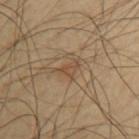The lesion was tiled from a total-body skin photograph and was not biopsied. The lesion's longest dimension is about 2.5 mm. A male subject aged 58 to 62. Automated image analysis of the tile measured a footprint of about 3 mm² and a shape-asymmetry score of about 0.5 (0 = symmetric). The lesion is located on the left upper arm. A roughly 15 mm field-of-view crop from a total-body skin photograph.